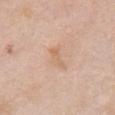biopsy status: total-body-photography surveillance lesion; no biopsy
imaging modality: total-body-photography crop, ~15 mm field of view
patient: female, roughly 60 years of age
diameter: about 3 mm
site: the chest